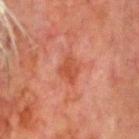lesion size: about 2.5 mm | body site: the head or neck | acquisition: 15 mm crop, total-body photography | subject: male, aged around 80 | automated metrics: an area of roughly 5.5 mm² and an outline eccentricity of about 0.5 (0 = round, 1 = elongated); a border-irregularity rating of about 2.5/10, a color-variation rating of about 2/10, and peripheral color asymmetry of about 1.